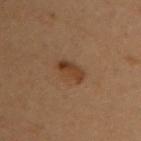Assessment: The lesion was tiled from a total-body skin photograph and was not biopsied. Clinical summary: This image is a 15 mm lesion crop taken from a total-body photograph. The tile uses cross-polarized illumination. A female patient aged 48 to 52. The lesion is on the chest.